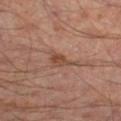Impression:
Captured during whole-body skin photography for melanoma surveillance; the lesion was not biopsied.
Clinical summary:
A region of skin cropped from a whole-body photographic capture, roughly 15 mm wide. The lesion's longest dimension is about 3.5 mm. This is a cross-polarized tile. A male patient, aged around 65. From the right thigh.